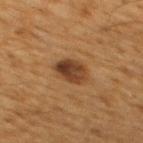Q: Was a biopsy performed?
A: total-body-photography surveillance lesion; no biopsy
Q: What are the patient's age and sex?
A: male, in their 60s
Q: Automated lesion metrics?
A: a footprint of about 8 mm², an eccentricity of roughly 0.6, and a symmetry-axis asymmetry near 0.15; internal color variation of about 6 on a 0–10 scale and a peripheral color-asymmetry measure near 2; a classifier nevus-likeness of about 80/100 and lesion-presence confidence of about 100/100
Q: What is the imaging modality?
A: 15 mm crop, total-body photography
Q: What is the anatomic site?
A: the back